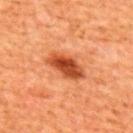notes: total-body-photography surveillance lesion; no biopsy
acquisition: total-body-photography crop, ~15 mm field of view
TBP lesion metrics: an outline eccentricity of about 0.85 (0 = round, 1 = elongated); a lesion color around L≈48 a*≈34 b*≈42 in CIELAB, a lesion–skin lightness drop of about 16, and a lesion-to-skin contrast of about 10.5 (normalized; higher = more distinct); an automated nevus-likeness rating near 95 out of 100 and lesion-presence confidence of about 100/100
patient: female, in their mid-50s
lesion size: ≈5 mm
location: the back
lighting: cross-polarized illumination